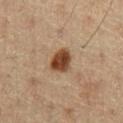Context: A male patient, in their 60s. A lesion tile, about 15 mm wide, cut from a 3D total-body photograph. The lesion's longest dimension is about 3.5 mm. The lesion-visualizer software estimated a lesion area of about 7 mm², an outline eccentricity of about 0.65 (0 = round, 1 = elongated), and two-axis asymmetry of about 0.2. And it measured a lesion-to-skin contrast of about 12 (normalized; higher = more distinct). It also reported a classifier nevus-likeness of about 100/100 and lesion-presence confidence of about 100/100.